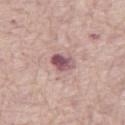Captured during whole-body skin photography for melanoma surveillance; the lesion was not biopsied. A roughly 15 mm field-of-view crop from a total-body skin photograph. The lesion is located on the right thigh. The tile uses white-light illumination. About 3 mm across. The subject is a female roughly 70 years of age.